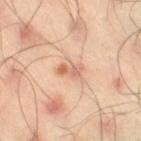Impression: The lesion was photographed on a routine skin check and not biopsied; there is no pathology result. Image and clinical context: Cropped from a whole-body photographic skin survey; the tile spans about 15 mm. An algorithmic analysis of the crop reported a nevus-likeness score of about 40/100 and a lesion-detection confidence of about 100/100. A male patient in their mid- to late 40s. Captured under cross-polarized illumination. Located on the right thigh.